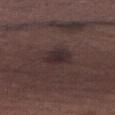{"image": {"source": "total-body photography crop", "field_of_view_mm": 15}, "patient": {"sex": "male", "age_approx": 70}, "site": "abdomen", "lighting": "white-light", "lesion_size": {"long_diameter_mm_approx": 3.5}, "automated_metrics": {"color_variation_0_10": 3.0}}